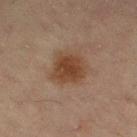Part of a total-body skin-imaging series; this lesion was reviewed on a skin check and was not flagged for biopsy. The recorded lesion diameter is about 4 mm. A female patient, aged 53 to 57. An algorithmic analysis of the crop reported a lesion area of about 12 mm², a shape eccentricity near 0.35, and a shape-asymmetry score of about 0.2 (0 = symmetric). The analysis additionally found an average lesion color of about L≈36 a*≈17 b*≈27 (CIELAB), about 9 CIELAB-L* units darker than the surrounding skin, and a lesion-to-skin contrast of about 9 (normalized; higher = more distinct). And it measured a classifier nevus-likeness of about 95/100 and a detector confidence of about 100 out of 100 that the crop contains a lesion. This image is a 15 mm lesion crop taken from a total-body photograph. This is a cross-polarized tile. Located on the left thigh.